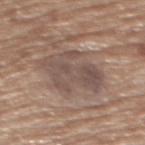The lesion was tiled from a total-body skin photograph and was not biopsied. Captured under white-light illumination. The recorded lesion diameter is about 7 mm. A female patient in their 70s. Cropped from a whole-body photographic skin survey; the tile spans about 15 mm. Automated image analysis of the tile measured an outline eccentricity of about 0.8 (0 = round, 1 = elongated). And it measured a mean CIELAB color near L≈50 a*≈14 b*≈22, a lesion–skin lightness drop of about 8, and a normalized border contrast of about 6.5. It also reported border irregularity of about 6 on a 0–10 scale, a within-lesion color-variation index near 4.5/10, and radial color variation of about 1.5. The analysis additionally found lesion-presence confidence of about 80/100. From the upper back.